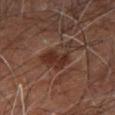Clinical impression: Captured during whole-body skin photography for melanoma surveillance; the lesion was not biopsied. Acquisition and patient details: A male subject approximately 60 years of age. An algorithmic analysis of the crop reported radial color variation of about 1. On the right leg. A 15 mm close-up tile from a total-body photography series done for melanoma screening. The tile uses cross-polarized illumination. Approximately 5 mm at its widest.